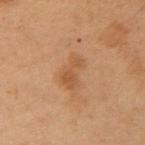No biopsy was performed on this lesion — it was imaged during a full skin examination and was not determined to be concerning.